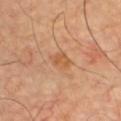Q: Automated lesion metrics?
A: an area of roughly 4 mm²; a lesion color around L≈56 a*≈23 b*≈38 in CIELAB, a lesion–skin lightness drop of about 7, and a normalized lesion–skin contrast near 6; internal color variation of about 2 on a 0–10 scale and a peripheral color-asymmetry measure near 0.5
Q: How large is the lesion?
A: ≈2.5 mm
Q: Illumination type?
A: cross-polarized
Q: What are the patient's age and sex?
A: male, in their mid- to late 60s
Q: How was this image acquired?
A: ~15 mm tile from a whole-body skin photo
Q: Where on the body is the lesion?
A: the chest